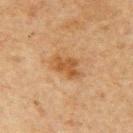biopsy status = no biopsy performed (imaged during a skin exam) | tile lighting = cross-polarized illumination | anatomic site = the arm | diameter = ~4 mm (longest diameter) | subject = male, aged approximately 65 | image = ~15 mm crop, total-body skin-cancer survey.